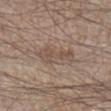notes: catalogued during a skin exam; not biopsied
lesion size: ~4.5 mm (longest diameter)
automated lesion analysis: a footprint of about 7 mm², an eccentricity of roughly 0.9, and two-axis asymmetry of about 0.35; an average lesion color of about L≈50 a*≈14 b*≈24 (CIELAB) and a lesion-to-skin contrast of about 5.5 (normalized; higher = more distinct); a nevus-likeness score of about 0/100 and a lesion-detection confidence of about 100/100
patient: male, aged approximately 45
anatomic site: the leg
acquisition: 15 mm crop, total-body photography
illumination: white-light illumination